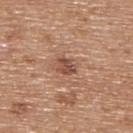follow-up: no biopsy performed (imaged during a skin exam)
location: the upper back
subject: male, aged around 65
acquisition: ~15 mm tile from a whole-body skin photo
image-analysis metrics: an average lesion color of about L≈49 a*≈22 b*≈28 (CIELAB), roughly 11 lightness units darker than nearby skin, and a normalized border contrast of about 8; a classifier nevus-likeness of about 65/100 and a detector confidence of about 100 out of 100 that the crop contains a lesion
size: ~2.5 mm (longest diameter)